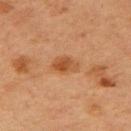{
  "biopsy_status": "not biopsied; imaged during a skin examination",
  "lighting": "cross-polarized",
  "site": "arm",
  "image": {
    "source": "total-body photography crop",
    "field_of_view_mm": 15
  },
  "automated_metrics": {
    "area_mm2_approx": 6.0,
    "eccentricity": 0.65,
    "shape_asymmetry": 0.25,
    "cielab_L": 44,
    "cielab_a": 21,
    "cielab_b": 34,
    "vs_skin_darker_L": 8.0,
    "vs_skin_contrast_norm": 7.0,
    "border_irregularity_0_10": 2.5,
    "color_variation_0_10": 4.5
  },
  "patient": {
    "sex": "female",
    "age_approx": 40
  }
}